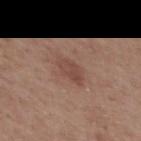  biopsy_status: not biopsied; imaged during a skin examination
  lesion_size:
    long_diameter_mm_approx: 3.0
  image:
    source: total-body photography crop
    field_of_view_mm: 15
  patient:
    sex: female
    age_approx: 50
  site: back
  lighting: white-light